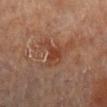notes = imaged on a skin check; not biopsied | image-analysis metrics = an automated nevus-likeness rating near 0 out of 100 | subject = female, aged 78 to 82 | imaging modality = ~15 mm tile from a whole-body skin photo | size = ~3.5 mm (longest diameter) | site = the leg | illumination = cross-polarized.